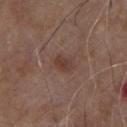Recorded during total-body skin imaging; not selected for excision or biopsy. A male subject, aged approximately 80. A lesion tile, about 15 mm wide, cut from a 3D total-body photograph. The lesion is on the chest. The total-body-photography lesion software estimated a lesion area of about 3.5 mm² and an outline eccentricity of about 0.8 (0 = round, 1 = elongated). Imaged with white-light lighting.